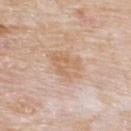Recorded during total-body skin imaging; not selected for excision or biopsy. The tile uses white-light illumination. A region of skin cropped from a whole-body photographic capture, roughly 15 mm wide. From the back. Approximately 4.5 mm at its widest. A male subject aged approximately 75.